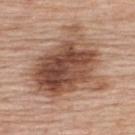Captured during whole-body skin photography for melanoma surveillance; the lesion was not biopsied.
The patient is a female aged around 65.
A close-up tile cropped from a whole-body skin photograph, about 15 mm across.
Automated image analysis of the tile measured an average lesion color of about L≈50 a*≈20 b*≈29 (CIELAB), roughly 15 lightness units darker than nearby skin, and a normalized border contrast of about 10. It also reported a border-irregularity index near 4.5/10 and a color-variation rating of about 9/10.
The lesion is located on the upper back.
Imaged with white-light lighting.
Measured at roughly 8.5 mm in maximum diameter.